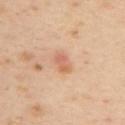<lesion>
<biopsy_status>not biopsied; imaged during a skin examination</biopsy_status>
<image>
  <source>total-body photography crop</source>
  <field_of_view_mm>15</field_of_view_mm>
</image>
<site>upper back</site>
<patient>
  <sex>female</sex>
  <age_approx>40</age_approx>
</patient>
</lesion>